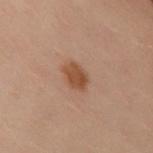Background: The lesion is located on the lower back. The lesion's longest dimension is about 3 mm. Cropped from a whole-body photographic skin survey; the tile spans about 15 mm. This is a cross-polarized tile. A female subject about 60 years old.